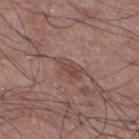workup: catalogued during a skin exam; not biopsied | subject: male, in their 60s | automated lesion analysis: a lesion area of about 6 mm²; an average lesion color of about L≈46 a*≈19 b*≈23 (CIELAB), a lesion–skin lightness drop of about 8, and a lesion-to-skin contrast of about 6 (normalized; higher = more distinct) | location: the left lower leg | image: total-body-photography crop, ~15 mm field of view | illumination: white-light.